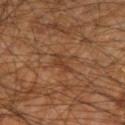Recorded during total-body skin imaging; not selected for excision or biopsy.